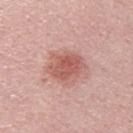lesion size = ~4 mm (longest diameter)
acquisition = ~15 mm tile from a whole-body skin photo
subject = male, about 30 years old